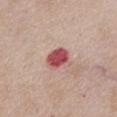Q: Was a biopsy performed?
A: imaged on a skin check; not biopsied
Q: What is the anatomic site?
A: the chest
Q: What lighting was used for the tile?
A: white-light
Q: Lesion size?
A: about 3 mm
Q: How was this image acquired?
A: ~15 mm crop, total-body skin-cancer survey
Q: Automated lesion metrics?
A: a footprint of about 7 mm², a shape eccentricity near 0.35, and two-axis asymmetry of about 0.2; a mean CIELAB color near L≈52 a*≈31 b*≈22; a border-irregularity rating of about 2/10 and a peripheral color-asymmetry measure near 1.5; a nevus-likeness score of about 0/100 and a lesion-detection confidence of about 100/100
Q: What are the patient's age and sex?
A: female, aged around 75